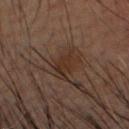No biopsy was performed on this lesion — it was imaged during a full skin examination and was not determined to be concerning. The lesion is on the head or neck. The tile uses cross-polarized illumination. Cropped from a total-body skin-imaging series; the visible field is about 15 mm. Approximately 2.5 mm at its widest. The patient is a male aged 48 to 52. Automated tile analysis of the lesion measured border irregularity of about 2.5 on a 0–10 scale. And it measured an automated nevus-likeness rating near 5 out of 100 and a detector confidence of about 70 out of 100 that the crop contains a lesion.